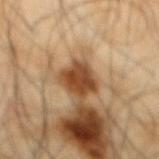Impression:
No biopsy was performed on this lesion — it was imaged during a full skin examination and was not determined to be concerning.
Context:
A male subject about 65 years old. A lesion tile, about 15 mm wide, cut from a 3D total-body photograph. On the mid back.